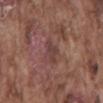Q: Is there a histopathology result?
A: no biopsy performed (imaged during a skin exam)
Q: How large is the lesion?
A: ~2.5 mm (longest diameter)
Q: What are the patient's age and sex?
A: male, roughly 75 years of age
Q: How was this image acquired?
A: ~15 mm tile from a whole-body skin photo
Q: Automated lesion metrics?
A: a lesion area of about 3 mm², a shape eccentricity near 0.8, and a symmetry-axis asymmetry near 0.45; border irregularity of about 5 on a 0–10 scale and radial color variation of about 0.5
Q: What lighting was used for the tile?
A: white-light illumination
Q: Lesion location?
A: the mid back